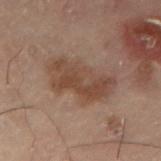{"biopsy_status": "not biopsied; imaged during a skin examination", "automated_metrics": {"border_irregularity_0_10": 4.0, "peripheral_color_asymmetry": 1.0}, "patient": {"sex": "female", "age_approx": 55}, "site": "lower back", "image": {"source": "total-body photography crop", "field_of_view_mm": 15}, "lighting": "cross-polarized"}